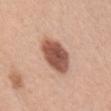notes = no biopsy performed (imaged during a skin exam); image source = 15 mm crop, total-body photography; tile lighting = white-light; body site = the front of the torso; subject = female, aged around 30.